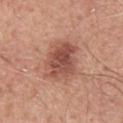– biopsy status · no biopsy performed (imaged during a skin exam)
– lesion diameter · about 5 mm
– TBP lesion metrics · a lesion color around L≈52 a*≈25 b*≈28 in CIELAB and about 11 CIELAB-L* units darker than the surrounding skin; a classifier nevus-likeness of about 70/100 and a detector confidence of about 100 out of 100 that the crop contains a lesion
– patient · male, aged 48–52
– image · total-body-photography crop, ~15 mm field of view
– illumination · white-light illumination
– body site · the chest